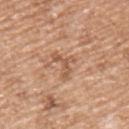Clinical impression: The lesion was tiled from a total-body skin photograph and was not biopsied. Clinical summary: From the left upper arm. A lesion tile, about 15 mm wide, cut from a 3D total-body photograph. A male subject, in their mid-50s. About 3 mm across.